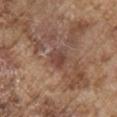Impression: Part of a total-body skin-imaging series; this lesion was reviewed on a skin check and was not flagged for biopsy. Clinical summary: A roughly 15 mm field-of-view crop from a total-body skin photograph. The patient is a male aged around 75. From the right upper arm.